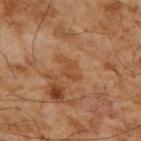<record>
  <biopsy_status>not biopsied; imaged during a skin examination</biopsy_status>
  <automated_metrics>
    <area_mm2_approx>3.5</area_mm2_approx>
    <eccentricity>0.8</eccentricity>
    <shape_asymmetry>0.4</shape_asymmetry>
    <border_irregularity_0_10>5.0</border_irregularity_0_10>
    <color_variation_0_10>0.0</color_variation_0_10>
  </automated_metrics>
  <image>
    <source>total-body photography crop</source>
    <field_of_view_mm>15</field_of_view_mm>
  </image>
  <site>right upper arm</site>
  <patient>
    <sex>male</sex>
    <age_approx>55</age_approx>
  </patient>
</record>